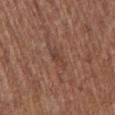The lesion was photographed on a routine skin check and not biopsied; there is no pathology result.
The lesion is located on the arm.
This image is a 15 mm lesion crop taken from a total-body photograph.
The lesion-visualizer software estimated a lesion area of about 3 mm², a shape eccentricity near 0.85, and a shape-asymmetry score of about 0.35 (0 = symmetric). The software also gave a border-irregularity index near 4/10, a within-lesion color-variation index near 1/10, and a peripheral color-asymmetry measure near 0.
About 3 mm across.
Imaged with white-light lighting.
A female patient, approximately 80 years of age.